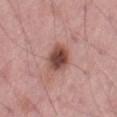Imaged during a routine full-body skin examination; the lesion was not biopsied and no histopathology is available. The lesion is located on the right thigh. The lesion-visualizer software estimated a lesion area of about 8 mm², an outline eccentricity of about 0.7 (0 = round, 1 = elongated), and a shape-asymmetry score of about 0.15 (0 = symmetric). The software also gave an average lesion color of about L≈47 a*≈24 b*≈24 (CIELAB), roughly 16 lightness units darker than nearby skin, and a normalized lesion–skin contrast near 11. It also reported a border-irregularity index near 1.5/10, a within-lesion color-variation index near 6/10, and a peripheral color-asymmetry measure near 1.5. The analysis additionally found a nevus-likeness score of about 95/100 and lesion-presence confidence of about 100/100. A male patient aged 53–57. About 3.5 mm across. Cropped from a total-body skin-imaging series; the visible field is about 15 mm.